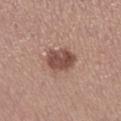Q: Was this lesion biopsied?
A: imaged on a skin check; not biopsied
Q: Where on the body is the lesion?
A: the leg
Q: Who is the patient?
A: female, in their mid- to late 30s
Q: How was this image acquired?
A: ~15 mm crop, total-body skin-cancer survey
Q: What is the lesion's diameter?
A: about 3.5 mm